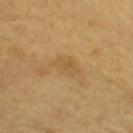No biopsy was performed on this lesion — it was imaged during a full skin examination and was not determined to be concerning.
The lesion is on the right upper arm.
A male subject, about 65 years old.
The recorded lesion diameter is about 3 mm.
A roughly 15 mm field-of-view crop from a total-body skin photograph.